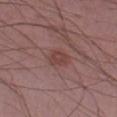workup: total-body-photography surveillance lesion; no biopsy | image source: 15 mm crop, total-body photography | body site: the left forearm | patient: male, aged 48–52 | TBP lesion metrics: a lesion area of about 5 mm², a shape eccentricity near 0.55, and a shape-asymmetry score of about 0.2 (0 = symmetric); a lesion–skin lightness drop of about 7 and a normalized lesion–skin contrast near 6.5; internal color variation of about 2 on a 0–10 scale; lesion-presence confidence of about 100/100.